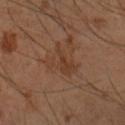Q: Is there a histopathology result?
A: imaged on a skin check; not biopsied
Q: What is the imaging modality?
A: ~15 mm tile from a whole-body skin photo
Q: What are the patient's age and sex?
A: male, aged around 65
Q: Lesion location?
A: the right forearm
Q: Automated lesion metrics?
A: a border-irregularity rating of about 8/10 and a peripheral color-asymmetry measure near 0.5; lesion-presence confidence of about 100/100